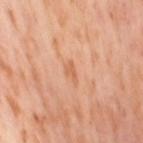Imaged during a routine full-body skin examination; the lesion was not biopsied and no histopathology is available.